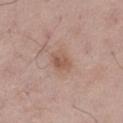From the left thigh. Longest diameter approximately 3 mm. The patient is a male roughly 50 years of age. A 15 mm crop from a total-body photograph taken for skin-cancer surveillance. An algorithmic analysis of the crop reported an area of roughly 5.5 mm² and an outline eccentricity of about 0.65 (0 = round, 1 = elongated). And it measured a border-irregularity index near 1.5/10. This is a white-light tile.